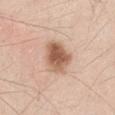Impression:
The lesion was tiled from a total-body skin photograph and was not biopsied.